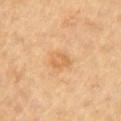Captured during whole-body skin photography for melanoma surveillance; the lesion was not biopsied. Imaged with cross-polarized lighting. A male subject approximately 85 years of age or older. A lesion tile, about 15 mm wide, cut from a 3D total-body photograph. The recorded lesion diameter is about 2.5 mm. The total-body-photography lesion software estimated a lesion area of about 4.5 mm², a shape eccentricity near 0.65, and a symmetry-axis asymmetry near 0.25. And it measured border irregularity of about 2 on a 0–10 scale and peripheral color asymmetry of about 0.5. And it measured a classifier nevus-likeness of about 15/100 and lesion-presence confidence of about 100/100. On the right upper arm.